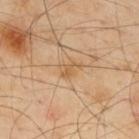Recorded during total-body skin imaging; not selected for excision or biopsy.
A male patient, aged 53 to 57.
A close-up tile cropped from a whole-body skin photograph, about 15 mm across.
The lesion is located on the upper back.